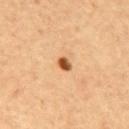| field | value |
|---|---|
| follow-up | catalogued during a skin exam; not biopsied |
| automated lesion analysis | an area of roughly 2.5 mm² and an outline eccentricity of about 0.7 (0 = round, 1 = elongated); an average lesion color of about L≈53 a*≈26 b*≈41 (CIELAB) and about 18 CIELAB-L* units darker than the surrounding skin |
| tile lighting | cross-polarized illumination |
| subject | male, aged around 60 |
| diameter | ≈2 mm |
| image source | total-body-photography crop, ~15 mm field of view |
| anatomic site | the mid back |